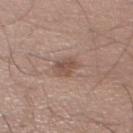Impression:
Captured during whole-body skin photography for melanoma surveillance; the lesion was not biopsied.
Clinical summary:
The recorded lesion diameter is about 3 mm. A male patient, aged approximately 40. The lesion is located on the left lower leg. Imaged with white-light lighting. A lesion tile, about 15 mm wide, cut from a 3D total-body photograph.